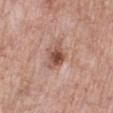Recorded during total-body skin imaging; not selected for excision or biopsy. A lesion tile, about 15 mm wide, cut from a 3D total-body photograph. The lesion's longest dimension is about 3 mm. The lesion is on the right lower leg. The total-body-photography lesion software estimated a border-irregularity rating of about 3/10, internal color variation of about 5.5 on a 0–10 scale, and peripheral color asymmetry of about 2. A female subject aged around 70. Captured under white-light illumination.